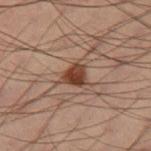notes: no biopsy performed (imaged during a skin exam); lighting: cross-polarized; patient: male, about 55 years old; body site: the right thigh; image source: ~15 mm crop, total-body skin-cancer survey; diameter: ≈3 mm.